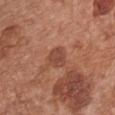Q: How was the tile lit?
A: white-light
Q: What is the anatomic site?
A: the front of the torso
Q: Patient demographics?
A: female, aged around 65
Q: What is the lesion's diameter?
A: ~2.5 mm (longest diameter)
Q: What is the imaging modality?
A: 15 mm crop, total-body photography